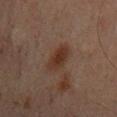The lesion was photographed on a routine skin check and not biopsied; there is no pathology result. Cropped from a whole-body photographic skin survey; the tile spans about 15 mm. Measured at roughly 4 mm in maximum diameter. Automated tile analysis of the lesion measured a shape eccentricity near 0.9 and a shape-asymmetry score of about 0.15 (0 = symmetric). The software also gave about 7 CIELAB-L* units darker than the surrounding skin and a normalized border contrast of about 9. The analysis additionally found border irregularity of about 2 on a 0–10 scale, a within-lesion color-variation index near 2.5/10, and peripheral color asymmetry of about 0.5. And it measured a classifier nevus-likeness of about 90/100 and a detector confidence of about 100 out of 100 that the crop contains a lesion. A male patient, aged 68–72. The lesion is located on the abdomen.